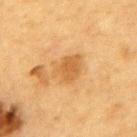| field | value |
|---|---|
| notes | no biopsy performed (imaged during a skin exam) |
| image source | ~15 mm crop, total-body skin-cancer survey |
| anatomic site | the back |
| tile lighting | cross-polarized |
| subject | male, about 85 years old or older |
| lesion diameter | ~3.5 mm (longest diameter) |